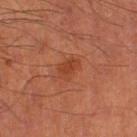Notes:
* biopsy status — imaged on a skin check; not biopsied
* image — ~15 mm crop, total-body skin-cancer survey
* lighting — cross-polarized
* image-analysis metrics — a mean CIELAB color near L≈33 a*≈24 b*≈29, about 6 CIELAB-L* units darker than the surrounding skin, and a normalized border contrast of about 6; a border-irregularity index near 2/10
* subject — male, approximately 65 years of age
* location — the leg